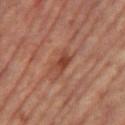notes: imaged on a skin check; not biopsied
patient: female, aged 63 to 67
body site: the left leg
tile lighting: cross-polarized illumination
image source: total-body-photography crop, ~15 mm field of view
automated lesion analysis: internal color variation of about 3 on a 0–10 scale
lesion size: about 3 mm